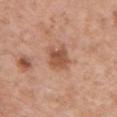Imaged during a routine full-body skin examination; the lesion was not biopsied and no histopathology is available. Cropped from a whole-body photographic skin survey; the tile spans about 15 mm. Automated image analysis of the tile measured a lesion area of about 6.5 mm², an outline eccentricity of about 0.55 (0 = round, 1 = elongated), and a symmetry-axis asymmetry near 0.25. It also reported a classifier nevus-likeness of about 70/100. The tile uses white-light illumination. A female subject approximately 50 years of age. On the chest.